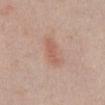Clinical summary: A male patient, roughly 40 years of age. The recorded lesion diameter is about 4 mm. This is a white-light tile. A roughly 15 mm field-of-view crop from a total-body skin photograph. From the abdomen.